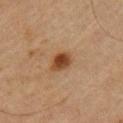Cropped from a total-body skin-imaging series; the visible field is about 15 mm.
A male patient, roughly 65 years of age.
From the left upper arm.